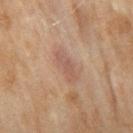Context: A female subject, aged approximately 60. The recorded lesion diameter is about 4 mm. A roughly 15 mm field-of-view crop from a total-body skin photograph. The lesion is on the right thigh. Imaged with cross-polarized lighting.